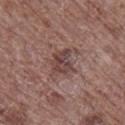Case summary:
* site: the right lower leg
* lighting: white-light
* diameter: ≈3 mm
* subject: male, in their 70s
* image: 15 mm crop, total-body photography